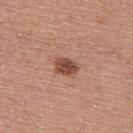follow-up = no biopsy performed (imaged during a skin exam).